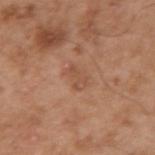The lesion was photographed on a routine skin check and not biopsied; there is no pathology result. A male patient, in their mid- to late 50s. A lesion tile, about 15 mm wide, cut from a 3D total-body photograph. The lesion is located on the right upper arm.